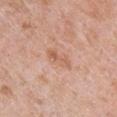image source = ~15 mm crop, total-body skin-cancer survey | body site = the chest | subject = female, aged approximately 40 | diameter = ~3.5 mm (longest diameter).